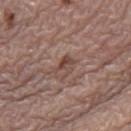Assessment: No biopsy was performed on this lesion — it was imaged during a full skin examination and was not determined to be concerning. Context: About 2.5 mm across. An algorithmic analysis of the crop reported a lesion area of about 3.5 mm², an outline eccentricity of about 0.65 (0 = round, 1 = elongated), and a symmetry-axis asymmetry near 0.4. The software also gave a classifier nevus-likeness of about 0/100 and a detector confidence of about 100 out of 100 that the crop contains a lesion. The patient is a male aged 68–72. Imaged with white-light lighting. A region of skin cropped from a whole-body photographic capture, roughly 15 mm wide. From the right thigh.